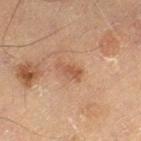The lesion was photographed on a routine skin check and not biopsied; there is no pathology result. The lesion is located on the leg. The patient is a male aged 68 to 72. A region of skin cropped from a whole-body photographic capture, roughly 15 mm wide. Longest diameter approximately 3 mm.